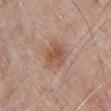{"biopsy_status": "not biopsied; imaged during a skin examination", "image": {"source": "total-body photography crop", "field_of_view_mm": 15}, "patient": {"sex": "male", "age_approx": 70}, "site": "head or neck", "lighting": "white-light"}